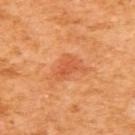follow-up: total-body-photography surveillance lesion; no biopsy | lesion diameter: about 3 mm | site: the upper back | tile lighting: cross-polarized | patient: female, in their mid- to late 50s | image-analysis metrics: a lesion-detection confidence of about 100/100 | image source: total-body-photography crop, ~15 mm field of view.